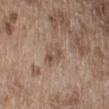Q: Is there a histopathology result?
A: no biopsy performed (imaged during a skin exam)